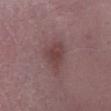– tile lighting · white-light
– body site · the right lower leg
– image · ~15 mm crop, total-body skin-cancer survey
– subject · male, aged 48 to 52
– diameter · ~4 mm (longest diameter)